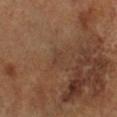Notes:
- follow-up — total-body-photography surveillance lesion; no biopsy
- lighting — cross-polarized
- location — the leg
- patient — male, about 65 years old
- image source — ~15 mm tile from a whole-body skin photo